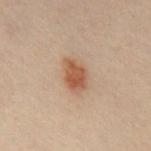notes = imaged on a skin check; not biopsied
tile lighting = cross-polarized
diameter = ~4 mm (longest diameter)
image source = ~15 mm tile from a whole-body skin photo
site = the abdomen
patient = male, aged approximately 50